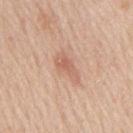Case summary:
* workup · no biopsy performed (imaged during a skin exam)